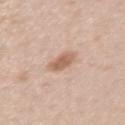This lesion was catalogued during total-body skin photography and was not selected for biopsy.